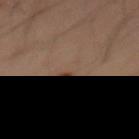notes: catalogued during a skin exam; not biopsied
site: the abdomen
image source: ~15 mm tile from a whole-body skin photo
lighting: cross-polarized
automated metrics: an area of roughly 3 mm², an outline eccentricity of about 0.75 (0 = round, 1 = elongated), and a symmetry-axis asymmetry near 0.7; a classifier nevus-likeness of about 0/100 and a lesion-detection confidence of about 35/100
patient: male, in their mid-30s